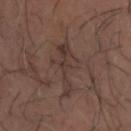Assessment:
Recorded during total-body skin imaging; not selected for excision or biopsy.
Background:
The recorded lesion diameter is about 5.5 mm. The lesion-visualizer software estimated a lesion area of about 9.5 mm² and two-axis asymmetry of about 0.6. The software also gave about 6 CIELAB-L* units darker than the surrounding skin. A male patient roughly 65 years of age. A roughly 15 mm field-of-view crop from a total-body skin photograph. On the head or neck.